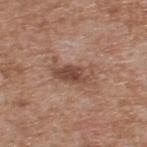biopsy status: no biopsy performed (imaged during a skin exam)
size: ~5 mm (longest diameter)
tile lighting: white-light
location: the upper back
subject: male, aged around 65
image: ~15 mm tile from a whole-body skin photo
automated lesion analysis: a mean CIELAB color near L≈48 a*≈20 b*≈27, roughly 10 lightness units darker than nearby skin, and a normalized lesion–skin contrast near 7.5; a border-irregularity rating of about 5.5/10, a color-variation rating of about 5.5/10, and radial color variation of about 2; an automated nevus-likeness rating near 25 out of 100 and lesion-presence confidence of about 100/100